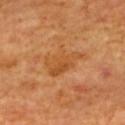<tbp_lesion>
  <biopsy_status>not biopsied; imaged during a skin examination</biopsy_status>
  <image>
    <source>total-body photography crop</source>
    <field_of_view_mm>15</field_of_view_mm>
  </image>
  <patient>
    <sex>male</sex>
    <age_approx>65</age_approx>
  </patient>
  <automated_metrics>
    <area_mm2_approx>10.0</area_mm2_approx>
    <shape_asymmetry>0.4</shape_asymmetry>
    <cielab_L>52</cielab_L>
    <cielab_a>26</cielab_a>
    <cielab_b>43</cielab_b>
    <vs_skin_contrast_norm>6.0</vs_skin_contrast_norm>
  </automated_metrics>
  <lesion_size>
    <long_diameter_mm_approx>5.0</long_diameter_mm_approx>
  </lesion_size>
  <lighting>cross-polarized</lighting>
  <site>upper back</site>
</tbp_lesion>